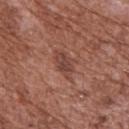Clinical impression:
No biopsy was performed on this lesion — it was imaged during a full skin examination and was not determined to be concerning.
Image and clinical context:
The tile uses white-light illumination. A male subject, in their mid- to late 70s. A 15 mm close-up extracted from a 3D total-body photography capture. Measured at roughly 3.5 mm in maximum diameter. On the upper back. An algorithmic analysis of the crop reported a lesion area of about 5.5 mm², an eccentricity of roughly 0.8, and a shape-asymmetry score of about 0.2 (0 = symmetric). And it measured about 9 CIELAB-L* units darker than the surrounding skin and a normalized lesion–skin contrast near 7.